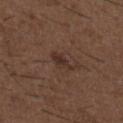notes=no biopsy performed (imaged during a skin exam) | subject=male, in their 50s | acquisition=~15 mm tile from a whole-body skin photo | anatomic site=the upper back | tile lighting=white-light | lesion size=about 2.5 mm.